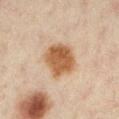patient: female, aged around 45 | image: ~15 mm crop, total-body skin-cancer survey | body site: the left lower leg | tile lighting: cross-polarized | lesion diameter: ~4.5 mm (longest diameter).